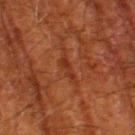Recorded during total-body skin imaging; not selected for excision or biopsy.
The tile uses cross-polarized illumination.
The lesion is located on the left thigh.
Approximately 3 mm at its widest.
A 15 mm close-up tile from a total-body photography series done for melanoma screening.
A male patient aged 78 to 82.